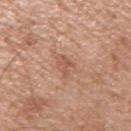Recorded during total-body skin imaging; not selected for excision or biopsy.
A close-up tile cropped from a whole-body skin photograph, about 15 mm across.
The lesion is located on the right upper arm.
A male subject aged approximately 65.
Imaged with white-light lighting.
Measured at roughly 3 mm in maximum diameter.
The lesion-visualizer software estimated a footprint of about 3.5 mm² and a shape eccentricity near 0.8. It also reported an average lesion color of about L≈56 a*≈22 b*≈31 (CIELAB), about 8 CIELAB-L* units darker than the surrounding skin, and a lesion-to-skin contrast of about 5.5 (normalized; higher = more distinct).